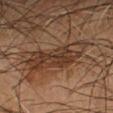{"image": {"source": "total-body photography crop", "field_of_view_mm": 15}, "patient": {"sex": "male", "age_approx": 55}, "site": "left forearm", "lighting": "cross-polarized", "lesion_size": {"long_diameter_mm_approx": 8.5}}